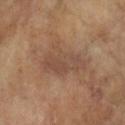Clinical impression:
Part of a total-body skin-imaging series; this lesion was reviewed on a skin check and was not flagged for biopsy.
Background:
The subject is a female in their mid-70s. Imaged with cross-polarized lighting. This image is a 15 mm lesion crop taken from a total-body photograph. The recorded lesion diameter is about 5.5 mm. From the left forearm.